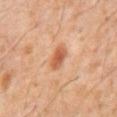Q: Is there a histopathology result?
A: catalogued during a skin exam; not biopsied
Q: What is the anatomic site?
A: the front of the torso
Q: Illumination type?
A: cross-polarized
Q: Patient demographics?
A: male, in their 60s
Q: What kind of image is this?
A: total-body-photography crop, ~15 mm field of view